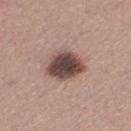Captured during whole-body skin photography for melanoma surveillance; the lesion was not biopsied. Imaged with white-light lighting. The lesion is on the left thigh. Cropped from a whole-body photographic skin survey; the tile spans about 15 mm. A female subject, in their 30s.